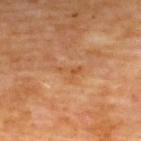biopsy status: catalogued during a skin exam; not biopsied
anatomic site: the upper back
TBP lesion metrics: a lesion area of about 2.5 mm² and an eccentricity of roughly 0.9; an average lesion color of about L≈53 a*≈24 b*≈40 (CIELAB), about 7 CIELAB-L* units darker than the surrounding skin, and a normalized border contrast of about 5.5; a border-irregularity rating of about 8.5/10, a within-lesion color-variation index near 0/10, and peripheral color asymmetry of about 0; a nevus-likeness score of about 0/100 and lesion-presence confidence of about 100/100
acquisition: ~15 mm crop, total-body skin-cancer survey
lighting: cross-polarized
patient: male, about 70 years old
lesion size: ≈3 mm